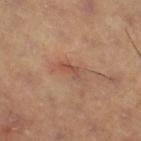biopsy status = no biopsy performed (imaged during a skin exam) | illumination = cross-polarized | diameter = ~3 mm (longest diameter) | image = ~15 mm crop, total-body skin-cancer survey | automated lesion analysis = a lesion color around L≈50 a*≈22 b*≈29 in CIELAB, a lesion–skin lightness drop of about 7, and a normalized lesion–skin contrast near 5.5; border irregularity of about 4.5 on a 0–10 scale, a within-lesion color-variation index near 0/10, and a peripheral color-asymmetry measure near 0; a nevus-likeness score of about 0/100 and a lesion-detection confidence of about 100/100 | subject = female, aged approximately 40 | site = the right thigh.